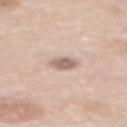Part of a total-body skin-imaging series; this lesion was reviewed on a skin check and was not flagged for biopsy. The lesion is located on the upper back. This image is a 15 mm lesion crop taken from a total-body photograph. A female patient aged 58 to 62.